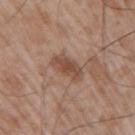Imaged during a routine full-body skin examination; the lesion was not biopsied and no histopathology is available. A 15 mm close-up tile from a total-body photography series done for melanoma screening. From the arm. The patient is a male roughly 50 years of age.